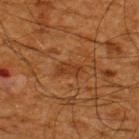Q: Was a biopsy performed?
A: imaged on a skin check; not biopsied
Q: Who is the patient?
A: male, aged around 65
Q: Automated lesion metrics?
A: an area of roughly 3.5 mm², an eccentricity of roughly 0.9, and a symmetry-axis asymmetry near 0.45; internal color variation of about 1.5 on a 0–10 scale and radial color variation of about 0.5
Q: How was the tile lit?
A: cross-polarized
Q: Lesion location?
A: the upper back
Q: How was this image acquired?
A: ~15 mm crop, total-body skin-cancer survey